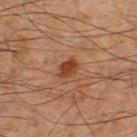Impression: Imaged during a routine full-body skin examination; the lesion was not biopsied and no histopathology is available. Clinical summary: Captured under cross-polarized illumination. A male subject roughly 60 years of age. A 15 mm close-up tile from a total-body photography series done for melanoma screening. Approximately 2.5 mm at its widest. On the right thigh.